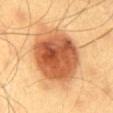The lesion was photographed on a routine skin check and not biopsied; there is no pathology result.
Located on the mid back.
A male patient in their mid- to late 40s.
A 15 mm close-up extracted from a 3D total-body photography capture.